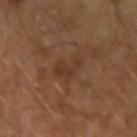The lesion was photographed on a routine skin check and not biopsied; there is no pathology result.
This is a cross-polarized tile.
The recorded lesion diameter is about 3.5 mm.
Cropped from a total-body skin-imaging series; the visible field is about 15 mm.
The patient is a male about 65 years old.